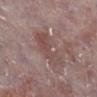Assessment:
The lesion was photographed on a routine skin check and not biopsied; there is no pathology result.
Acquisition and patient details:
On the leg. About 5 mm across. The subject is a male roughly 75 years of age. Cropped from a total-body skin-imaging series; the visible field is about 15 mm.